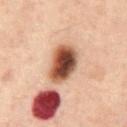Clinical impression: Recorded during total-body skin imaging; not selected for excision or biopsy. Context: This is a cross-polarized tile. A male subject, aged approximately 60. The total-body-photography lesion software estimated roughly 18 lightness units darker than nearby skin and a normalized lesion–skin contrast near 14. And it measured a classifier nevus-likeness of about 100/100 and lesion-presence confidence of about 100/100. On the abdomen. A lesion tile, about 15 mm wide, cut from a 3D total-body photograph. About 4.5 mm across.